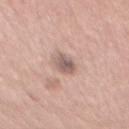Q: Is there a histopathology result?
A: imaged on a skin check; not biopsied
Q: How was the tile lit?
A: white-light
Q: What is the imaging modality?
A: total-body-photography crop, ~15 mm field of view
Q: What did automated image analysis measure?
A: roughly 13 lightness units darker than nearby skin and a normalized lesion–skin contrast near 8.5
Q: What is the anatomic site?
A: the mid back
Q: Lesion size?
A: about 3 mm
Q: Who is the patient?
A: male, in their mid- to late 40s